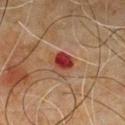Q: Was a biopsy performed?
A: catalogued during a skin exam; not biopsied
Q: What did automated image analysis measure?
A: a lesion area of about 6 mm², a shape eccentricity near 0.35, and two-axis asymmetry of about 0.25; about 12 CIELAB-L* units darker than the surrounding skin and a normalized border contrast of about 10.5; border irregularity of about 2.5 on a 0–10 scale and a within-lesion color-variation index near 6/10
Q: How was the tile lit?
A: cross-polarized
Q: What are the patient's age and sex?
A: male, in their 60s
Q: Lesion location?
A: the chest
Q: How large is the lesion?
A: ~3 mm (longest diameter)
Q: How was this image acquired?
A: 15 mm crop, total-body photography